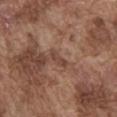Notes:
– workup · catalogued during a skin exam; not biopsied
– body site · the abdomen
– tile lighting · white-light illumination
– diameter · about 3 mm
– subject · male, in their mid-70s
– acquisition · 15 mm crop, total-body photography